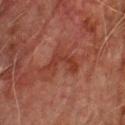This lesion was catalogued during total-body skin photography and was not selected for biopsy. Longest diameter approximately 4 mm. A male patient aged 73 to 77. The lesion is on the chest. A 15 mm close-up extracted from a 3D total-body photography capture.